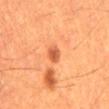This lesion was catalogued during total-body skin photography and was not selected for biopsy.
Imaged with cross-polarized lighting.
The patient is a male about 60 years old.
A 15 mm close-up extracted from a 3D total-body photography capture.
Longest diameter approximately 2.5 mm.
Automated image analysis of the tile measured a lesion area of about 3 mm², an eccentricity of roughly 0.8, and a shape-asymmetry score of about 0.25 (0 = symmetric). It also reported a mean CIELAB color near L≈55 a*≈31 b*≈40, about 12 CIELAB-L* units darker than the surrounding skin, and a normalized border contrast of about 7.5. The software also gave an automated nevus-likeness rating near 70 out of 100.
The lesion is located on the mid back.